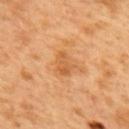The lesion was photographed on a routine skin check and not biopsied; there is no pathology result. The lesion is located on the upper back. The recorded lesion diameter is about 3 mm. A male patient, aged 48–52. This image is a 15 mm lesion crop taken from a total-body photograph. The lesion-visualizer software estimated a lesion color around L≈59 a*≈25 b*≈43 in CIELAB, roughly 8 lightness units darker than nearby skin, and a normalized lesion–skin contrast near 5.5. And it measured a color-variation rating of about 3/10 and peripheral color asymmetry of about 1.